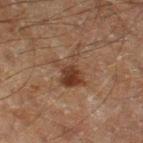Clinical impression: Captured during whole-body skin photography for melanoma surveillance; the lesion was not biopsied. Clinical summary: The lesion is on the right thigh. The lesion's longest dimension is about 5 mm. Cropped from a total-body skin-imaging series; the visible field is about 15 mm. A male patient aged around 60. This is a cross-polarized tile.